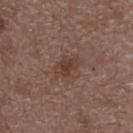{"biopsy_status": "not biopsied; imaged during a skin examination", "patient": {"sex": "female", "age_approx": 65}, "site": "upper back", "image": {"source": "total-body photography crop", "field_of_view_mm": 15}, "lighting": "white-light", "lesion_size": {"long_diameter_mm_approx": 2.5}}